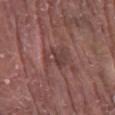  biopsy_status: not biopsied; imaged during a skin examination
  lesion_size:
    long_diameter_mm_approx: 3.5
  patient:
    sex: male
    age_approx: 80
  site: lower back
  lighting: white-light
  image:
    source: total-body photography crop
    field_of_view_mm: 15